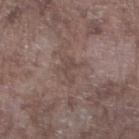Clinical summary:
A male subject, approximately 75 years of age. Cropped from a total-body skin-imaging series; the visible field is about 15 mm. About 3 mm across. The lesion is located on the leg.